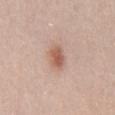Findings:
– follow-up: catalogued during a skin exam; not biopsied
– body site: the mid back
– patient: male, in their mid-20s
– automated metrics: an eccentricity of roughly 0.7 and two-axis asymmetry of about 0.2; a mean CIELAB color near L≈59 a*≈22 b*≈28 and a lesion-to-skin contrast of about 7.5 (normalized; higher = more distinct)
– acquisition: 15 mm crop, total-body photography
– illumination: white-light